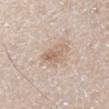notes: no biopsy performed (imaged during a skin exam)
body site: the right lower leg
lighting: white-light illumination
automated lesion analysis: a footprint of about 7 mm² and a shape-asymmetry score of about 0.4 (0 = symmetric); a lesion color around L≈64 a*≈15 b*≈28 in CIELAB, roughly 9 lightness units darker than nearby skin, and a lesion-to-skin contrast of about 6 (normalized; higher = more distinct); internal color variation of about 4 on a 0–10 scale and radial color variation of about 1.5; a classifier nevus-likeness of about 0/100 and lesion-presence confidence of about 100/100
size: ~4 mm (longest diameter)
image source: 15 mm crop, total-body photography
subject: female, approximately 45 years of age